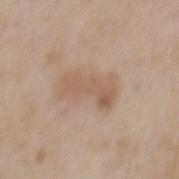The lesion was tiled from a total-body skin photograph and was not biopsied.
The recorded lesion diameter is about 5 mm.
A male patient, in their 50s.
On the mid back.
A 15 mm close-up extracted from a 3D total-body photography capture.
Captured under white-light illumination.